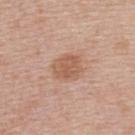– workup · catalogued during a skin exam; not biopsied
– image source · 15 mm crop, total-body photography
– site · the back
– TBP lesion metrics · a lesion area of about 9 mm², an eccentricity of roughly 0.55, and a shape-asymmetry score of about 0.2 (0 = symmetric); an average lesion color of about L≈58 a*≈21 b*≈31 (CIELAB), about 9 CIELAB-L* units darker than the surrounding skin, and a normalized lesion–skin contrast near 6.5; a border-irregularity rating of about 2/10, a color-variation rating of about 2/10, and a peripheral color-asymmetry measure near 1; a nevus-likeness score of about 40/100 and a detector confidence of about 100 out of 100 that the crop contains a lesion
– patient · female, aged 48–52